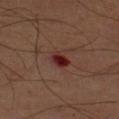| feature | finding |
|---|---|
| follow-up | imaged on a skin check; not biopsied |
| acquisition | ~15 mm crop, total-body skin-cancer survey |
| size | ≈2.5 mm |
| patient | male, aged 43 to 47 |
| body site | the left upper arm |